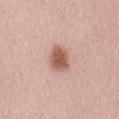{
  "biopsy_status": "not biopsied; imaged during a skin examination",
  "lesion_size": {
    "long_diameter_mm_approx": 3.5
  },
  "lighting": "white-light",
  "image": {
    "source": "total-body photography crop",
    "field_of_view_mm": 15
  },
  "site": "abdomen",
  "patient": {
    "sex": "female",
    "age_approx": 25
  },
  "automated_metrics": {
    "cielab_L": 57,
    "cielab_a": 23,
    "cielab_b": 28,
    "vs_skin_darker_L": 14.0,
    "vs_skin_contrast_norm": 9.5
  }
}